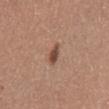The lesion was photographed on a routine skin check and not biopsied; there is no pathology result. The lesion is located on the abdomen. A 15 mm close-up extracted from a 3D total-body photography capture. A female subject aged 48–52. The tile uses white-light illumination. Longest diameter approximately 3 mm. The total-body-photography lesion software estimated a mean CIELAB color near L≈48 a*≈20 b*≈28 and a lesion–skin lightness drop of about 12. The software also gave a border-irregularity index near 2.5/10, a color-variation rating of about 3.5/10, and radial color variation of about 1. The software also gave a classifier nevus-likeness of about 90/100 and lesion-presence confidence of about 100/100.